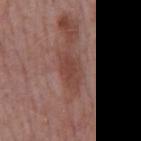notes: imaged on a skin check; not biopsied
size: ≈4.5 mm
tile lighting: white-light illumination
site: the back
TBP lesion metrics: a lesion color around L≈43 a*≈23 b*≈24 in CIELAB, roughly 8 lightness units darker than nearby skin, and a normalized border contrast of about 6.5; border irregularity of about 3 on a 0–10 scale, a color-variation rating of about 2/10, and a peripheral color-asymmetry measure near 0.5; a nevus-likeness score of about 0/100 and lesion-presence confidence of about 100/100
subject: male, about 75 years old
acquisition: 15 mm crop, total-body photography